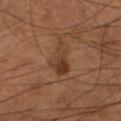Captured during whole-body skin photography for melanoma surveillance; the lesion was not biopsied.
Located on the left lower leg.
This is a cross-polarized tile.
A male subject aged 53–57.
A lesion tile, about 15 mm wide, cut from a 3D total-body photograph.
Longest diameter approximately 4.5 mm.